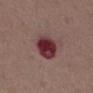  biopsy_status: not biopsied; imaged during a skin examination
  site: chest
  automated_metrics:
    cielab_L: 34
    cielab_a: 27
    cielab_b: 16
    vs_skin_darker_L: 16.0
    nevus_likeness_0_100: 0
    lesion_detection_confidence_0_100: 100
  patient:
    sex: male
    age_approx: 50
  lesion_size:
    long_diameter_mm_approx: 4.5
  image:
    source: total-body photography crop
    field_of_view_mm: 15